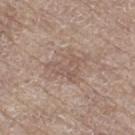Q: Was this lesion biopsied?
A: imaged on a skin check; not biopsied
Q: What is the imaging modality?
A: ~15 mm tile from a whole-body skin photo
Q: Patient demographics?
A: male, aged 68 to 72
Q: How large is the lesion?
A: ≈4.5 mm
Q: Automated lesion metrics?
A: an area of roughly 9 mm² and a symmetry-axis asymmetry near 0.5; an average lesion color of about L≈55 a*≈15 b*≈24 (CIELAB), about 7 CIELAB-L* units darker than the surrounding skin, and a lesion-to-skin contrast of about 5 (normalized; higher = more distinct); a nevus-likeness score of about 0/100
Q: Where on the body is the lesion?
A: the right thigh